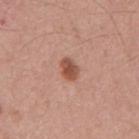<case>
  <biopsy_status>not biopsied; imaged during a skin examination</biopsy_status>
  <image>
    <source>total-body photography crop</source>
    <field_of_view_mm>15</field_of_view_mm>
  </image>
  <automated_metrics>
    <area_mm2_approx>4.0</area_mm2_approx>
    <shape_asymmetry>0.2</shape_asymmetry>
    <cielab_L>51</cielab_L>
    <cielab_a>25</cielab_a>
    <cielab_b>29</cielab_b>
    <vs_skin_darker_L>13.0</vs_skin_darker_L>
    <vs_skin_contrast_norm>9.0</vs_skin_contrast_norm>
    <border_irregularity_0_10>1.5</border_irregularity_0_10>
    <color_variation_0_10>2.5</color_variation_0_10>
    <peripheral_color_asymmetry>1.0</peripheral_color_asymmetry>
    <nevus_likeness_0_100>95</nevus_likeness_0_100>
  </automated_metrics>
  <lesion_size>
    <long_diameter_mm_approx>2.5</long_diameter_mm_approx>
  </lesion_size>
  <lighting>white-light</lighting>
  <site>mid back</site>
  <patient>
    <sex>male</sex>
    <age_approx>55</age_approx>
  </patient>
</case>